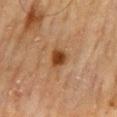Notes:
* workup: total-body-photography surveillance lesion; no biopsy
* location: the mid back
* lighting: cross-polarized illumination
* automated metrics: an area of roughly 5 mm², an outline eccentricity of about 0.65 (0 = round, 1 = elongated), and a symmetry-axis asymmetry near 0.25; border irregularity of about 2.5 on a 0–10 scale and a peripheral color-asymmetry measure near 0.5
* size: ≈3 mm
* acquisition: ~15 mm tile from a whole-body skin photo
* patient: male, approximately 70 years of age